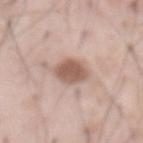Assessment: The lesion was tiled from a total-body skin photograph and was not biopsied. Background: A lesion tile, about 15 mm wide, cut from a 3D total-body photograph. Captured under white-light illumination. On the abdomen. A male subject approximately 55 years of age.